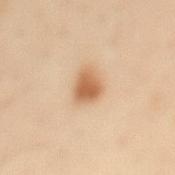Clinical impression:
This lesion was catalogued during total-body skin photography and was not selected for biopsy.
Background:
A male patient aged approximately 55. From the mid back. Cropped from a whole-body photographic skin survey; the tile spans about 15 mm. The tile uses cross-polarized illumination. About 3 mm across.